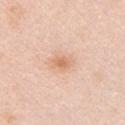{"biopsy_status": "not biopsied; imaged during a skin examination", "lesion_size": {"long_diameter_mm_approx": 2.5}, "lighting": "white-light", "site": "chest", "image": {"source": "total-body photography crop", "field_of_view_mm": 15}, "patient": {"sex": "female", "age_approx": 40}}